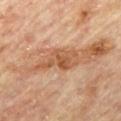No biopsy was performed on this lesion — it was imaged during a full skin examination and was not determined to be concerning.
Imaged with cross-polarized lighting.
Cropped from a whole-body photographic skin survey; the tile spans about 15 mm.
About 5.5 mm across.
On the back.
A male patient, aged around 85 or older.